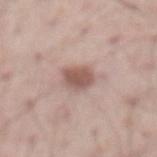body site: the abdomen; subject: male, aged approximately 65; lighting: white-light illumination; lesion diameter: ≈3 mm; image: ~15 mm tile from a whole-body skin photo.